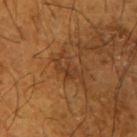Recorded during total-body skin imaging; not selected for excision or biopsy. This is a cross-polarized tile. The patient is a male in their mid-60s. The lesion is on the right upper arm. A region of skin cropped from a whole-body photographic capture, roughly 15 mm wide. The lesion-visualizer software estimated roughly 6 lightness units darker than nearby skin and a lesion-to-skin contrast of about 5.5 (normalized; higher = more distinct). The software also gave a border-irregularity index near 5/10, internal color variation of about 3.5 on a 0–10 scale, and peripheral color asymmetry of about 1.5. It also reported a classifier nevus-likeness of about 0/100 and a detector confidence of about 95 out of 100 that the crop contains a lesion. The recorded lesion diameter is about 4 mm.